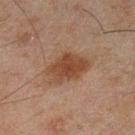{"biopsy_status": "not biopsied; imaged during a skin examination", "automated_metrics": {"color_variation_0_10": 2.5, "peripheral_color_asymmetry": 0.5}, "image": {"source": "total-body photography crop", "field_of_view_mm": 15}, "site": "left lower leg", "lesion_size": {"long_diameter_mm_approx": 4.5}, "lighting": "cross-polarized", "patient": {"sex": "male", "age_approx": 45}}